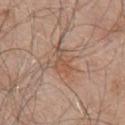patient: male, aged approximately 80
imaging modality: ~15 mm crop, total-body skin-cancer survey
tile lighting: white-light illumination
lesion size: ≈3.5 mm
automated lesion analysis: border irregularity of about 6.5 on a 0–10 scale, internal color variation of about 2.5 on a 0–10 scale, and peripheral color asymmetry of about 1; a classifier nevus-likeness of about 0/100 and a lesion-detection confidence of about 100/100
anatomic site: the front of the torso